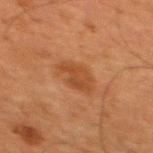Recorded during total-body skin imaging; not selected for excision or biopsy.
The patient is a male aged 63 to 67.
Measured at roughly 4 mm in maximum diameter.
Imaged with cross-polarized lighting.
A 15 mm close-up tile from a total-body photography series done for melanoma screening.
Located on the mid back.